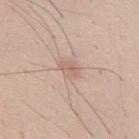Assessment:
This lesion was catalogued during total-body skin photography and was not selected for biopsy.
Image and clinical context:
Imaged with white-light lighting. The lesion is on the mid back. A male subject, about 35 years old. A 15 mm close-up tile from a total-body photography series done for melanoma screening. About 2.5 mm across.